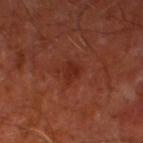Assessment: The lesion was tiled from a total-body skin photograph and was not biopsied. Background: From the leg. This image is a 15 mm lesion crop taken from a total-body photograph. A male patient in their mid- to late 60s.